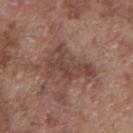No biopsy was performed on this lesion — it was imaged during a full skin examination and was not determined to be concerning. A roughly 15 mm field-of-view crop from a total-body skin photograph. The patient is a male aged 73–77. Located on the chest. Automated tile analysis of the lesion measured a peripheral color-asymmetry measure near 1. Longest diameter approximately 5.5 mm. The tile uses white-light illumination.